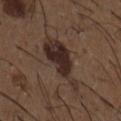follow-up: no biopsy performed (imaged during a skin exam) | automated lesion analysis: a footprint of about 15 mm², a shape eccentricity near 0.9, and two-axis asymmetry of about 0.4; a border-irregularity rating of about 5.5/10 and a peripheral color-asymmetry measure near 1.5; a nevus-likeness score of about 85/100 | lesion diameter: ≈7.5 mm | image: ~15 mm crop, total-body skin-cancer survey | patient: male, aged 48 to 52 | site: the chest.